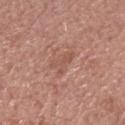<record>
<biopsy_status>not biopsied; imaged during a skin examination</biopsy_status>
<site>head or neck</site>
<patient>
  <sex>male</sex>
  <age_approx>60</age_approx>
</patient>
<image>
  <source>total-body photography crop</source>
  <field_of_view_mm>15</field_of_view_mm>
</image>
</record>